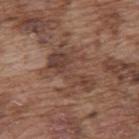Notes:
* follow-up — catalogued during a skin exam; not biopsied
* size — ~6 mm (longest diameter)
* imaging modality — ~15 mm tile from a whole-body skin photo
* TBP lesion metrics — a border-irregularity index near 8/10, a color-variation rating of about 3/10, and radial color variation of about 0.5
* tile lighting — white-light illumination
* subject — male, in their mid- to late 70s
* body site — the upper back